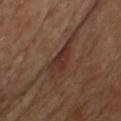  biopsy_status: not biopsied; imaged during a skin examination
  lighting: cross-polarized
  image:
    source: total-body photography crop
    field_of_view_mm: 15
  site: front of the torso